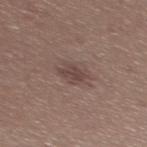Imaged during a routine full-body skin examination; the lesion was not biopsied and no histopathology is available.
A region of skin cropped from a whole-body photographic capture, roughly 15 mm wide.
The total-body-photography lesion software estimated a mean CIELAB color near L≈43 a*≈16 b*≈19, a lesion–skin lightness drop of about 8, and a lesion-to-skin contrast of about 7 (normalized; higher = more distinct). The software also gave a border-irregularity index near 2.5/10, a color-variation rating of about 1.5/10, and peripheral color asymmetry of about 0.5. The software also gave a classifier nevus-likeness of about 10/100 and lesion-presence confidence of about 100/100.
The lesion is on the upper back.
Captured under white-light illumination.
A female patient, in their mid- to late 20s.